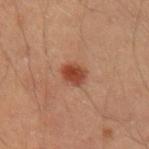Imaged during a routine full-body skin examination; the lesion was not biopsied and no histopathology is available. The subject is a male aged approximately 40. Approximately 3 mm at its widest. A roughly 15 mm field-of-view crop from a total-body skin photograph. Captured under cross-polarized illumination. The lesion is located on the left upper arm.